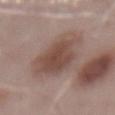| key | value |
|---|---|
| workup | catalogued during a skin exam; not biopsied |
| lesion diameter | ~7 mm (longest diameter) |
| subject | male, in their mid-50s |
| anatomic site | the mid back |
| illumination | white-light illumination |
| acquisition | ~15 mm tile from a whole-body skin photo |